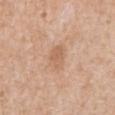biopsy status — total-body-photography surveillance lesion; no biopsy | size — about 3 mm | body site — the abdomen | patient — male, about 60 years old | image — ~15 mm tile from a whole-body skin photo | lighting — white-light.